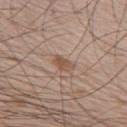Captured during whole-body skin photography for melanoma surveillance; the lesion was not biopsied. Measured at roughly 2.5 mm in maximum diameter. A male subject, approximately 65 years of age. On the right upper arm. The tile uses white-light illumination. Cropped from a total-body skin-imaging series; the visible field is about 15 mm.